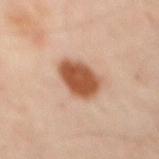From the mid back. A patient approximately 55 years of age. A roughly 15 mm field-of-view crop from a total-body skin photograph.